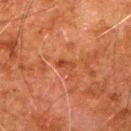Captured during whole-body skin photography for melanoma surveillance; the lesion was not biopsied.
A male patient, aged approximately 80.
From the left upper arm.
A lesion tile, about 15 mm wide, cut from a 3D total-body photograph.